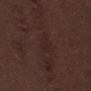The lesion was tiled from a total-body skin photograph and was not biopsied.
A male patient, aged around 70.
A 15 mm close-up extracted from a 3D total-body photography capture.
The lesion is located on the back.